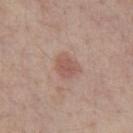biopsy_status: not biopsied; imaged during a skin examination
image:
  source: total-body photography crop
  field_of_view_mm: 15
patient:
  sex: male
  age_approx: 55
site: front of the torso
lighting: white-light